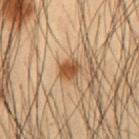No biopsy was performed on this lesion — it was imaged during a full skin examination and was not determined to be concerning. This is a cross-polarized tile. This image is a 15 mm lesion crop taken from a total-body photograph. A male subject, in their mid-50s. The lesion is on the front of the torso.